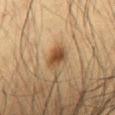Q: Is there a histopathology result?
A: imaged on a skin check; not biopsied
Q: What are the patient's age and sex?
A: male, roughly 35 years of age
Q: Where on the body is the lesion?
A: the abdomen
Q: What did automated image analysis measure?
A: an area of roughly 4.5 mm² and a shape-asymmetry score of about 0.25 (0 = symmetric); a lesion color around L≈44 a*≈18 b*≈34 in CIELAB and about 12 CIELAB-L* units darker than the surrounding skin; a border-irregularity rating of about 2/10, internal color variation of about 3 on a 0–10 scale, and a peripheral color-asymmetry measure near 1
Q: How was the tile lit?
A: cross-polarized illumination
Q: Lesion size?
A: ≈2.5 mm
Q: What is the imaging modality?
A: 15 mm crop, total-body photography